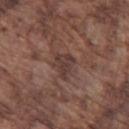Impression:
The lesion was photographed on a routine skin check and not biopsied; there is no pathology result.
Acquisition and patient details:
The patient is a male aged approximately 75. Longest diameter approximately 3 mm. Located on the left thigh. A 15 mm close-up tile from a total-body photography series done for melanoma screening.